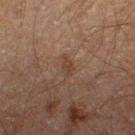Notes:
* biopsy status — no biopsy performed (imaged during a skin exam)
* imaging modality — total-body-photography crop, ~15 mm field of view
* patient — male, aged approximately 60
* site — the leg